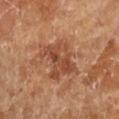| field | value |
|---|---|
| notes | no biopsy performed (imaged during a skin exam) |
| image-analysis metrics | border irregularity of about 5 on a 0–10 scale, internal color variation of about 5.5 on a 0–10 scale, and peripheral color asymmetry of about 2; a classifier nevus-likeness of about 0/100 and lesion-presence confidence of about 100/100 |
| patient | female, aged approximately 70 |
| body site | the right forearm |
| diameter | ≈5 mm |
| imaging modality | ~15 mm crop, total-body skin-cancer survey |
| tile lighting | cross-polarized illumination |